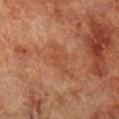Assessment:
The lesion was photographed on a routine skin check and not biopsied; there is no pathology result.
Context:
A close-up tile cropped from a whole-body skin photograph, about 15 mm across. A male patient in their 70s. Automated tile analysis of the lesion measured an area of roughly 6 mm² and a shape-asymmetry score of about 0.3 (0 = symmetric). The analysis additionally found internal color variation of about 1.5 on a 0–10 scale and radial color variation of about 0.5. The analysis additionally found a classifier nevus-likeness of about 0/100 and a detector confidence of about 100 out of 100 that the crop contains a lesion. The tile uses cross-polarized illumination. Approximately 4 mm at its widest. The lesion is located on the right lower leg.